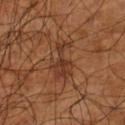{"biopsy_status": "not biopsied; imaged during a skin examination", "image": {"source": "total-body photography crop", "field_of_view_mm": 15}, "site": "left lower leg", "patient": {"sex": "male", "age_approx": 60}, "lesion_size": {"long_diameter_mm_approx": 5.0}, "lighting": "cross-polarized"}